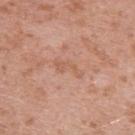Captured during whole-body skin photography for melanoma surveillance; the lesion was not biopsied.
On the left upper arm.
The lesion's longest dimension is about 3.5 mm.
A male patient aged around 40.
A roughly 15 mm field-of-view crop from a total-body skin photograph.
Captured under white-light illumination.